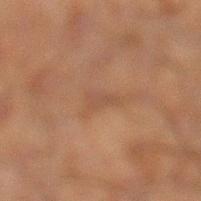Assessment:
Part of a total-body skin-imaging series; this lesion was reviewed on a skin check and was not flagged for biopsy.
Context:
The lesion is located on the leg. A region of skin cropped from a whole-body photographic capture, roughly 15 mm wide. An algorithmic analysis of the crop reported a footprint of about 2.5 mm² and a symmetry-axis asymmetry near 0.4. It also reported border irregularity of about 4 on a 0–10 scale and peripheral color asymmetry of about 0. The software also gave a detector confidence of about 85 out of 100 that the crop contains a lesion. A male subject, aged around 50. Imaged with cross-polarized lighting.